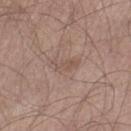Recorded during total-body skin imaging; not selected for excision or biopsy.
This is a white-light tile.
A region of skin cropped from a whole-body photographic capture, roughly 15 mm wide.
An algorithmic analysis of the crop reported a footprint of about 4 mm², an eccentricity of roughly 0.85, and a shape-asymmetry score of about 0.4 (0 = symmetric). The software also gave a mean CIELAB color near L≈52 a*≈16 b*≈25 and a lesion–skin lightness drop of about 6. And it measured a nevus-likeness score of about 0/100 and a detector confidence of about 100 out of 100 that the crop contains a lesion.
Located on the right lower leg.
The subject is a male approximately 60 years of age.
Longest diameter approximately 3 mm.